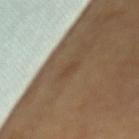The lesion was photographed on a routine skin check and not biopsied; there is no pathology result.
Approximately 2.5 mm at its widest.
A female patient, aged 58 to 62.
Imaged with cross-polarized lighting.
A 15 mm close-up tile from a total-body photography series done for melanoma screening.
From the mid back.